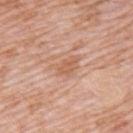Findings:
* notes · catalogued during a skin exam; not biopsied
* patient · male, aged 48 to 52
* site · the left upper arm
* tile lighting · white-light illumination
* acquisition · 15 mm crop, total-body photography
* TBP lesion metrics · an area of roughly 6.5 mm² and a shape eccentricity near 0.75; an average lesion color of about L≈61 a*≈22 b*≈32 (CIELAB), about 7 CIELAB-L* units darker than the surrounding skin, and a lesion-to-skin contrast of about 5.5 (normalized; higher = more distinct)
* diameter · about 4 mm